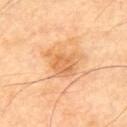The lesion was photographed on a routine skin check and not biopsied; there is no pathology result.
A male patient roughly 60 years of age.
This is a cross-polarized tile.
A close-up tile cropped from a whole-body skin photograph, about 15 mm across.
The recorded lesion diameter is about 4 mm.
On the upper back.
The total-body-photography lesion software estimated an area of roughly 9 mm² and a shape-asymmetry score of about 0.35 (0 = symmetric). And it measured a border-irregularity rating of about 4/10, internal color variation of about 3 on a 0–10 scale, and a peripheral color-asymmetry measure near 1. It also reported a classifier nevus-likeness of about 0/100 and lesion-presence confidence of about 100/100.